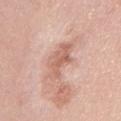Findings:
• biopsy status — total-body-photography surveillance lesion; no biopsy
• location — the chest
• patient — female, in their 40s
• lesion diameter — about 5.5 mm
• image source — total-body-photography crop, ~15 mm field of view